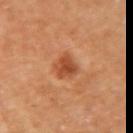On the left upper arm. A 15 mm close-up extracted from a 3D total-body photography capture. The subject is a male approximately 65 years of age.